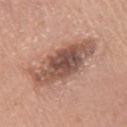The lesion was photographed on a routine skin check and not biopsied; there is no pathology result. The lesion is located on the chest. The patient is a female roughly 60 years of age. A roughly 15 mm field-of-view crop from a total-body skin photograph. The tile uses white-light illumination. The lesion-visualizer software estimated about 14 CIELAB-L* units darker than the surrounding skin and a normalized lesion–skin contrast near 9.